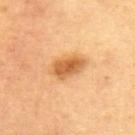This lesion was catalogued during total-body skin photography and was not selected for biopsy. The lesion is located on the upper back. A male subject in their mid- to late 50s. A 15 mm close-up tile from a total-body photography series done for melanoma screening.